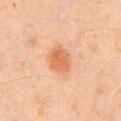Captured during whole-body skin photography for melanoma surveillance; the lesion was not biopsied.